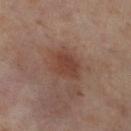<tbp_lesion>
  <biopsy_status>not biopsied; imaged during a skin examination</biopsy_status>
  <lesion_size>
    <long_diameter_mm_approx>4.5</long_diameter_mm_approx>
  </lesion_size>
  <site>leg</site>
  <patient>
    <sex>female</sex>
    <age_approx>55</age_approx>
  </patient>
  <lighting>cross-polarized</lighting>
  <image>
    <source>total-body photography crop</source>
    <field_of_view_mm>15</field_of_view_mm>
  </image>
</tbp_lesion>